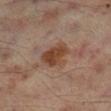follow-up=imaged on a skin check; not biopsied | body site=the right lower leg | lighting=cross-polarized illumination | size=≈4 mm | acquisition=~15 mm tile from a whole-body skin photo | automated lesion analysis=a lesion area of about 10 mm² | subject=male, in their mid-60s.